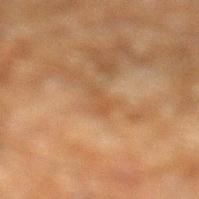Notes:
• biopsy status — total-body-photography surveillance lesion; no biopsy
• site — the left lower leg
• illumination — cross-polarized
• imaging modality — ~15 mm crop, total-body skin-cancer survey
• subject — male, aged approximately 60
• size — ~2.5 mm (longest diameter)